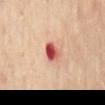The lesion was tiled from a total-body skin photograph and was not biopsied.
Captured under cross-polarized illumination.
A female patient, in their 60s.
The lesion is located on the back.
A 15 mm close-up tile from a total-body photography series done for melanoma screening.
The recorded lesion diameter is about 3 mm.